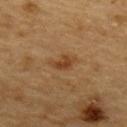* notes — total-body-photography surveillance lesion; no biopsy
* body site — the upper back
* subject — male, aged 83 or older
* tile lighting — cross-polarized
* image source — ~15 mm tile from a whole-body skin photo